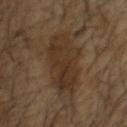Notes:
– notes — imaged on a skin check; not biopsied
– lesion size — ~7 mm (longest diameter)
– body site — the head or neck
– tile lighting — cross-polarized illumination
– patient — male, aged 53–57
– image — total-body-photography crop, ~15 mm field of view